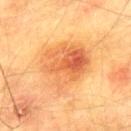No biopsy was performed on this lesion — it was imaged during a full skin examination and was not determined to be concerning. A close-up tile cropped from a whole-body skin photograph, about 15 mm across. The lesion-visualizer software estimated an outline eccentricity of about 0.65 (0 = round, 1 = elongated) and a symmetry-axis asymmetry near 0.25. The analysis additionally found a border-irregularity index near 2.5/10, a color-variation rating of about 9/10, and a peripheral color-asymmetry measure near 3. A male patient aged approximately 75. Located on the upper back.